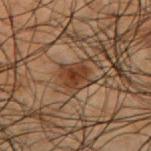Notes:
* site: the upper back
* lighting: cross-polarized
* lesion diameter: ~3 mm (longest diameter)
* image source: ~15 mm tile from a whole-body skin photo
* TBP lesion metrics: a shape eccentricity near 0.6 and a symmetry-axis asymmetry near 0.2; a mean CIELAB color near L≈28 a*≈17 b*≈25, roughly 8 lightness units darker than nearby skin, and a normalized lesion–skin contrast near 9; a border-irregularity rating of about 2/10, internal color variation of about 3.5 on a 0–10 scale, and a peripheral color-asymmetry measure near 1; an automated nevus-likeness rating near 70 out of 100 and a detector confidence of about 100 out of 100 that the crop contains a lesion
* subject: male, approximately 50 years of age